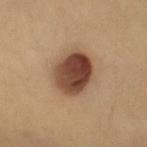biopsy status=catalogued during a skin exam; not biopsied
subject=female, approximately 35 years of age
image source=total-body-photography crop, ~15 mm field of view
site=the right upper arm
size=~5 mm (longest diameter)
tile lighting=cross-polarized illumination